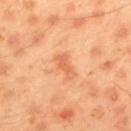• workup — imaged on a skin check; not biopsied
• lesion size — about 3 mm
• anatomic site — the back
• image source — ~15 mm crop, total-body skin-cancer survey
• patient — male, about 45 years old
• tile lighting — cross-polarized